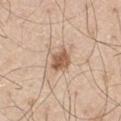| feature | finding |
|---|---|
| biopsy status | imaged on a skin check; not biopsied |
| location | the leg |
| subject | male, aged 68–72 |
| imaging modality | total-body-photography crop, ~15 mm field of view |
| automated metrics | a lesion area of about 5.5 mm², a shape eccentricity near 0.5, and two-axis asymmetry of about 0.25; an average lesion color of about L≈58 a*≈19 b*≈32 (CIELAB), roughly 13 lightness units darker than nearby skin, and a normalized border contrast of about 9; a nevus-likeness score of about 75/100 and a detector confidence of about 100 out of 100 that the crop contains a lesion |
| lesion diameter | ≈3 mm |